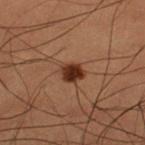The lesion was photographed on a routine skin check and not biopsied; there is no pathology result. An algorithmic analysis of the crop reported a lesion area of about 4.5 mm², an outline eccentricity of about 0.65 (0 = round, 1 = elongated), and two-axis asymmetry of about 0.15. And it measured a border-irregularity rating of about 1.5/10 and a peripheral color-asymmetry measure near 1. Measured at roughly 2.5 mm in maximum diameter. Captured under cross-polarized illumination. The lesion is on the left thigh. A male patient aged 48–52. A 15 mm close-up extracted from a 3D total-body photography capture.